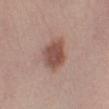A female patient, about 45 years old.
Located on the left lower leg.
Cropped from a total-body skin-imaging series; the visible field is about 15 mm.
The tile uses white-light illumination.
The lesion-visualizer software estimated a lesion area of about 11 mm², an eccentricity of roughly 0.6, and a shape-asymmetry score of about 0.2 (0 = symmetric). And it measured a border-irregularity rating of about 2/10, internal color variation of about 3 on a 0–10 scale, and a peripheral color-asymmetry measure near 1.
Longest diameter approximately 4 mm.